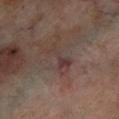Notes:
• workup: total-body-photography surveillance lesion; no biopsy
• patient: male, roughly 70 years of age
• image source: ~15 mm tile from a whole-body skin photo
• location: the right lower leg
• TBP lesion metrics: a lesion area of about 6 mm², an eccentricity of roughly 0.9, and a symmetry-axis asymmetry near 0.5; about 6 CIELAB-L* units darker than the surrounding skin and a lesion-to-skin contrast of about 6 (normalized; higher = more distinct); an automated nevus-likeness rating near 0 out of 100 and a lesion-detection confidence of about 50/100
• illumination: cross-polarized
• diameter: ≈4 mm